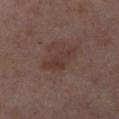Acquisition and patient details: Cropped from a total-body skin-imaging series; the visible field is about 15 mm. A female patient in their mid-50s. On the right lower leg. Longest diameter approximately 4 mm.